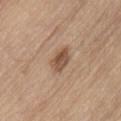body site: the lower back | image source: total-body-photography crop, ~15 mm field of view | subject: male, aged 68 to 72.